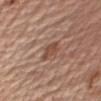| key | value |
|---|---|
| workup | total-body-photography surveillance lesion; no biopsy |
| tile lighting | white-light |
| image source | 15 mm crop, total-body photography |
| location | the right upper arm |
| subject | male, aged approximately 75 |
| automated lesion analysis | a classifier nevus-likeness of about 15/100 and a lesion-detection confidence of about 95/100 |
| lesion diameter | ≈3.5 mm |